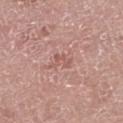No biopsy was performed on this lesion — it was imaged during a full skin examination and was not determined to be concerning. Approximately 3 mm at its widest. The lesion is on the left lower leg. Cropped from a whole-body photographic skin survey; the tile spans about 15 mm. The total-body-photography lesion software estimated a lesion area of about 3.5 mm² and a shape-asymmetry score of about 0.45 (0 = symmetric). The analysis additionally found border irregularity of about 5.5 on a 0–10 scale, a within-lesion color-variation index near 0/10, and peripheral color asymmetry of about 0. A male patient about 75 years old. The tile uses white-light illumination.